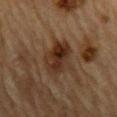| key | value |
|---|---|
| workup | catalogued during a skin exam; not biopsied |
| subject | male, aged approximately 85 or older |
| site | the mid back |
| image source | 15 mm crop, total-body photography |
| lesion size | ~5 mm (longest diameter) |
| illumination | cross-polarized illumination |